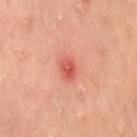This lesion was catalogued during total-body skin photography and was not selected for biopsy.
The lesion-visualizer software estimated a lesion color around L≈58 a*≈36 b*≈33 in CIELAB, roughly 12 lightness units darker than nearby skin, and a normalized lesion–skin contrast near 7.5.
The patient is a female aged approximately 35.
From the right thigh.
This is a cross-polarized tile.
Cropped from a whole-body photographic skin survey; the tile spans about 15 mm.